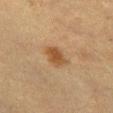Imaged during a routine full-body skin examination; the lesion was not biopsied and no histopathology is available. This is a cross-polarized tile. A lesion tile, about 15 mm wide, cut from a 3D total-body photograph. Automated image analysis of the tile measured a lesion area of about 6 mm² and a symmetry-axis asymmetry near 0.2. It also reported a mean CIELAB color near L≈43 a*≈17 b*≈33, about 9 CIELAB-L* units darker than the surrounding skin, and a lesion-to-skin contrast of about 8 (normalized; higher = more distinct). From the front of the torso. The patient is a female approximately 55 years of age. Longest diameter approximately 3 mm.